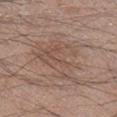Recorded during total-body skin imaging; not selected for excision or biopsy. A 15 mm crop from a total-body photograph taken for skin-cancer surveillance. The subject is a male approximately 20 years of age. Located on the right lower leg.